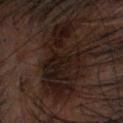The lesion was photographed on a routine skin check and not biopsied; there is no pathology result. Captured under cross-polarized illumination. About 8 mm across. Cropped from a whole-body photographic skin survey; the tile spans about 15 mm. A male subject aged 68 to 72. The lesion is on the head or neck. The lesion-visualizer software estimated a border-irregularity index near 6.5/10, internal color variation of about 4.5 on a 0–10 scale, and a peripheral color-asymmetry measure near 1.5.